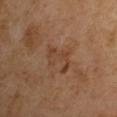Notes:
• notes · imaged on a skin check; not biopsied
• patient · male, approximately 65 years of age
• lighting · cross-polarized illumination
• acquisition · ~15 mm tile from a whole-body skin photo